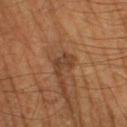Recorded during total-body skin imaging; not selected for excision or biopsy.
Located on the left lower leg.
Measured at roughly 2.5 mm in maximum diameter.
The lesion-visualizer software estimated an area of roughly 4 mm², a shape eccentricity near 0.7, and a symmetry-axis asymmetry near 0.35. It also reported a classifier nevus-likeness of about 0/100 and a lesion-detection confidence of about 100/100.
This is a cross-polarized tile.
A male subject, approximately 65 years of age.
A region of skin cropped from a whole-body photographic capture, roughly 15 mm wide.